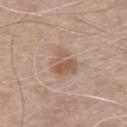  biopsy_status: not biopsied; imaged during a skin examination
  patient:
    sex: male
    age_approx: 65
  image:
    source: total-body photography crop
    field_of_view_mm: 15
  site: chest
  lesion_size:
    long_diameter_mm_approx: 3.5